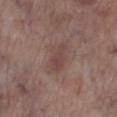The lesion was photographed on a routine skin check and not biopsied; there is no pathology result.
The lesion is on the leg.
A male subject approximately 70 years of age.
The total-body-photography lesion software estimated a footprint of about 5 mm², an outline eccentricity of about 0.9 (0 = round, 1 = elongated), and a shape-asymmetry score of about 0.3 (0 = symmetric). And it measured a mean CIELAB color near L≈43 a*≈19 b*≈19, about 7 CIELAB-L* units darker than the surrounding skin, and a normalized border contrast of about 6. The analysis additionally found a classifier nevus-likeness of about 0/100 and lesion-presence confidence of about 100/100.
A roughly 15 mm field-of-view crop from a total-body skin photograph.